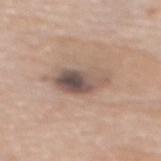This lesion was catalogued during total-body skin photography and was not selected for biopsy.
The tile uses white-light illumination.
A female patient, approximately 60 years of age.
On the mid back.
About 6 mm across.
This image is a 15 mm lesion crop taken from a total-body photograph.